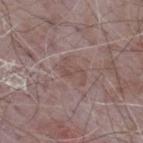The lesion was photographed on a routine skin check and not biopsied; there is no pathology result.